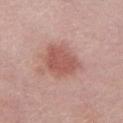Impression: The lesion was tiled from a total-body skin photograph and was not biopsied. Context: On the chest. A female patient in their 60s. About 4 mm across. Captured under white-light illumination. A region of skin cropped from a whole-body photographic capture, roughly 15 mm wide.